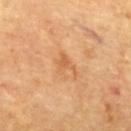This lesion was catalogued during total-body skin photography and was not selected for biopsy. The lesion is on the upper back. About 3 mm across. A 15 mm crop from a total-body photograph taken for skin-cancer surveillance. A subject aged around 60. Imaged with cross-polarized lighting.